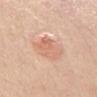Impression: The lesion was tiled from a total-body skin photograph and was not biopsied. Acquisition and patient details: From the chest. A female subject aged 53 to 57. The recorded lesion diameter is about 4 mm. A close-up tile cropped from a whole-body skin photograph, about 15 mm across.